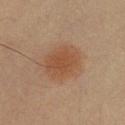Captured during whole-body skin photography for melanoma surveillance; the lesion was not biopsied. Measured at roughly 4.5 mm in maximum diameter. A 15 mm close-up tile from a total-body photography series done for melanoma screening. From the chest. The patient is a male in their mid-30s. An algorithmic analysis of the crop reported a footprint of about 15 mm², an outline eccentricity of about 0.45 (0 = round, 1 = elongated), and a symmetry-axis asymmetry near 0.1. The software also gave an average lesion color of about L≈44 a*≈18 b*≈29 (CIELAB), a lesion–skin lightness drop of about 7, and a normalized border contrast of about 6.5. It also reported a border-irregularity rating of about 1.5/10, a color-variation rating of about 2.5/10, and radial color variation of about 1.